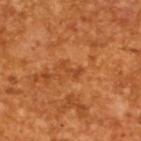Background:
This image is a 15 mm lesion crop taken from a total-body photograph. A male subject, about 65 years old. The total-body-photography lesion software estimated a footprint of about 2 mm² and an eccentricity of roughly 0.9. And it measured a mean CIELAB color near L≈45 a*≈29 b*≈42 and a normalized lesion–skin contrast near 5.5. Longest diameter approximately 2.5 mm.